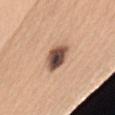Findings:
- workup: catalogued during a skin exam; not biopsied
- acquisition: 15 mm crop, total-body photography
- size: ≈3.5 mm
- automated lesion analysis: a footprint of about 8.5 mm² and an eccentricity of roughly 0.55; a lesion color around L≈50 a*≈18 b*≈26 in CIELAB, a lesion–skin lightness drop of about 20, and a lesion-to-skin contrast of about 13.5 (normalized; higher = more distinct); a classifier nevus-likeness of about 30/100 and a lesion-detection confidence of about 100/100
- subject: female, aged 58 to 62
- body site: the right upper arm
- illumination: white-light illumination